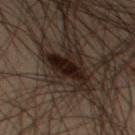Captured during whole-body skin photography for melanoma surveillance; the lesion was not biopsied. Automated tile analysis of the lesion measured an outline eccentricity of about 0.65 (0 = round, 1 = elongated) and a shape-asymmetry score of about 0.25 (0 = symmetric). The analysis additionally found a nevus-likeness score of about 100/100. A male patient, about 55 years old. The recorded lesion diameter is about 4 mm. A 15 mm crop from a total-body photograph taken for skin-cancer surveillance. The tile uses cross-polarized illumination. On the left thigh.